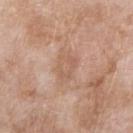Assessment: Imaged during a routine full-body skin examination; the lesion was not biopsied and no histopathology is available. Context: Automated tile analysis of the lesion measured a mean CIELAB color near L≈59 a*≈19 b*≈30, roughly 6 lightness units darker than nearby skin, and a lesion-to-skin contrast of about 4.5 (normalized; higher = more distinct). The software also gave a classifier nevus-likeness of about 0/100 and a lesion-detection confidence of about 100/100. Captured under white-light illumination. The lesion is on the right forearm. This image is a 15 mm lesion crop taken from a total-body photograph. A female patient aged 73 to 77.